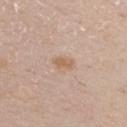This lesion was catalogued during total-body skin photography and was not selected for biopsy.
From the front of the torso.
A region of skin cropped from a whole-body photographic capture, roughly 15 mm wide.
The patient is a male approximately 60 years of age.
The recorded lesion diameter is about 2.5 mm.